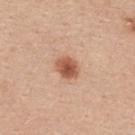<tbp_lesion>
  <biopsy_status>not biopsied; imaged during a skin examination</biopsy_status>
  <site>upper back</site>
  <patient>
    <sex>female</sex>
    <age_approx>40</age_approx>
  </patient>
  <image>
    <source>total-body photography crop</source>
    <field_of_view_mm>15</field_of_view_mm>
  </image>
  <lesion_size>
    <long_diameter_mm_approx>3.0</long_diameter_mm_approx>
  </lesion_size>
</tbp_lesion>